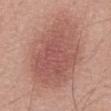Clinical impression: Part of a total-body skin-imaging series; this lesion was reviewed on a skin check and was not flagged for biopsy. Image and clinical context: A male patient aged 53–57. The tile uses white-light illumination. The lesion is on the abdomen. A 15 mm close-up extracted from a 3D total-body photography capture. Automated tile analysis of the lesion measured an area of roughly 48 mm². The analysis additionally found border irregularity of about 2 on a 0–10 scale, a color-variation rating of about 4/10, and radial color variation of about 1. The analysis additionally found a lesion-detection confidence of about 100/100.